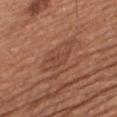The lesion was tiled from a total-body skin photograph and was not biopsied. The recorded lesion diameter is about 5 mm. A male subject approximately 65 years of age. A 15 mm crop from a total-body photograph taken for skin-cancer surveillance. Imaged with white-light lighting. An algorithmic analysis of the crop reported a mean CIELAB color near L≈45 a*≈23 b*≈30 and a normalized lesion–skin contrast near 5. The software also gave a color-variation rating of about 2/10. The lesion is located on the upper back.